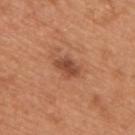Part of a total-body skin-imaging series; this lesion was reviewed on a skin check and was not flagged for biopsy. A male patient, aged around 65. The lesion is located on the right upper arm. A 15 mm close-up tile from a total-body photography series done for melanoma screening.